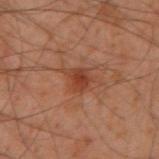Case summary:
– workup: catalogued during a skin exam; not biopsied
– subject: male, about 50 years old
– location: the left arm
– image-analysis metrics: an average lesion color of about L≈33 a*≈22 b*≈27 (CIELAB), roughly 8 lightness units darker than nearby skin, and a normalized lesion–skin contrast near 7; lesion-presence confidence of about 100/100
– illumination: cross-polarized
– acquisition: ~15 mm tile from a whole-body skin photo
– lesion diameter: ≈3 mm